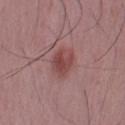Part of a total-body skin-imaging series; this lesion was reviewed on a skin check and was not flagged for biopsy. This image is a 15 mm lesion crop taken from a total-body photograph. This is a white-light tile. A male patient aged 48 to 52. From the left upper arm. Measured at roughly 4 mm in maximum diameter.